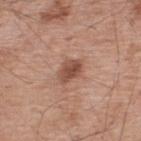Assessment: No biopsy was performed on this lesion — it was imaged during a full skin examination and was not determined to be concerning. Background: About 3 mm across. Located on the upper back. Cropped from a whole-body photographic skin survey; the tile spans about 15 mm. The tile uses white-light illumination. A male subject in their mid-50s.